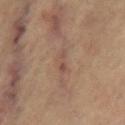workup — catalogued during a skin exam; not biopsied | acquisition — 15 mm crop, total-body photography | illumination — cross-polarized illumination | image-analysis metrics — a lesion area of about 3 mm², an eccentricity of roughly 0.95, and a shape-asymmetry score of about 0.4 (0 = symmetric); a lesion color around L≈49 a*≈18 b*≈25 in CIELAB and a lesion–skin lightness drop of about 7; border irregularity of about 4 on a 0–10 scale, internal color variation of about 0 on a 0–10 scale, and peripheral color asymmetry of about 0; an automated nevus-likeness rating near 0 out of 100 | subject — female, in their mid- to late 60s | site — the right thigh.